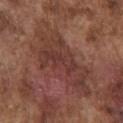Imaged during a routine full-body skin examination; the lesion was not biopsied and no histopathology is available. On the chest. A male patient about 75 years old. Cropped from a whole-body photographic skin survey; the tile spans about 15 mm.